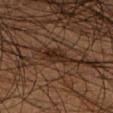Impression:
The lesion was photographed on a routine skin check and not biopsied; there is no pathology result.
Image and clinical context:
Located on the right lower leg. A male subject approximately 45 years of age. Captured under cross-polarized illumination. A 15 mm close-up extracted from a 3D total-body photography capture. An algorithmic analysis of the crop reported a lesion area of about 6 mm², an outline eccentricity of about 0.75 (0 = round, 1 = elongated), and a shape-asymmetry score of about 0.3 (0 = symmetric). And it measured an average lesion color of about L≈25 a*≈16 b*≈24 (CIELAB) and roughly 8 lightness units darker than nearby skin. It also reported a nevus-likeness score of about 75/100 and a lesion-detection confidence of about 95/100.